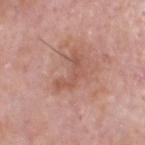Case summary:
* biopsy status · no biopsy performed (imaged during a skin exam)
* image · ~15 mm tile from a whole-body skin photo
* illumination · white-light illumination
* patient · male, roughly 70 years of age
* TBP lesion metrics · a footprint of about 7 mm², an eccentricity of roughly 0.85, and two-axis asymmetry of about 0.7; a border-irregularity index near 8.5/10, a within-lesion color-variation index near 2/10, and a peripheral color-asymmetry measure near 0.5
* lesion size · ~4.5 mm (longest diameter)
* site · the head or neck